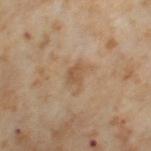Q: Was this lesion biopsied?
A: imaged on a skin check; not biopsied
Q: Who is the patient?
A: female, in their mid- to late 50s
Q: Lesion size?
A: ~2.5 mm (longest diameter)
Q: How was the tile lit?
A: cross-polarized illumination
Q: How was this image acquired?
A: ~15 mm crop, total-body skin-cancer survey
Q: Lesion location?
A: the leg